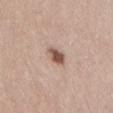biopsy status: imaged on a skin check; not biopsied
anatomic site: the back
patient: female, aged 33–37
image source: ~15 mm crop, total-body skin-cancer survey
lesion diameter: about 2.5 mm
image-analysis metrics: a lesion area of about 4 mm², a shape eccentricity near 0.65, and a shape-asymmetry score of about 0.25 (0 = symmetric)
tile lighting: white-light